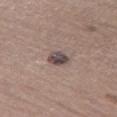This lesion was catalogued during total-body skin photography and was not selected for biopsy.
Measured at roughly 3 mm in maximum diameter.
Captured under white-light illumination.
From the leg.
The subject is a female approximately 65 years of age.
This image is a 15 mm lesion crop taken from a total-body photograph.